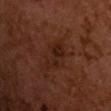Captured during whole-body skin photography for melanoma surveillance; the lesion was not biopsied.
Longest diameter approximately 4 mm.
From the upper back.
A lesion tile, about 15 mm wide, cut from a 3D total-body photograph.
Automated tile analysis of the lesion measured a lesion area of about 5.5 mm² and an eccentricity of roughly 0.9. It also reported a lesion–skin lightness drop of about 6 and a normalized lesion–skin contrast near 7. It also reported a nevus-likeness score of about 15/100.
A male subject, aged approximately 55.